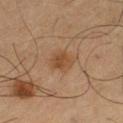notes: catalogued during a skin exam; not biopsied | acquisition: ~15 mm tile from a whole-body skin photo | diameter: ~3 mm (longest diameter) | site: the left thigh | patient: male, in their mid- to late 60s | illumination: cross-polarized | TBP lesion metrics: a lesion area of about 5 mm², an eccentricity of roughly 0.6, and a symmetry-axis asymmetry near 0.2; a lesion color around L≈37 a*≈16 b*≈28 in CIELAB, roughly 7 lightness units darker than nearby skin, and a normalized lesion–skin contrast near 7; a border-irregularity index near 2/10 and internal color variation of about 2 on a 0–10 scale; lesion-presence confidence of about 100/100.